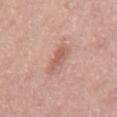biopsy_status: not biopsied; imaged during a skin examination
site: mid back
patient:
  sex: male
  age_approx: 55
lighting: white-light
image:
  source: total-body photography crop
  field_of_view_mm: 15